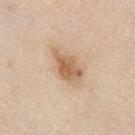body site: the front of the torso
acquisition: ~15 mm crop, total-body skin-cancer survey
patient: male, approximately 80 years of age